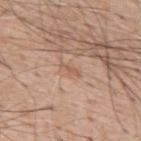Captured during whole-body skin photography for melanoma surveillance; the lesion was not biopsied. A 15 mm close-up extracted from a 3D total-body photography capture. A male patient aged 58–62. Located on the back. This is a white-light tile. The lesion's longest dimension is about 2.5 mm.